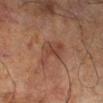Assessment: No biopsy was performed on this lesion — it was imaged during a full skin examination and was not determined to be concerning. Image and clinical context: Automated image analysis of the tile measured a classifier nevus-likeness of about 0/100 and a detector confidence of about 100 out of 100 that the crop contains a lesion. Measured at roughly 5 mm in maximum diameter. From the leg. Cropped from a total-body skin-imaging series; the visible field is about 15 mm. The tile uses cross-polarized illumination. The patient is a male aged approximately 70.